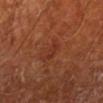Assessment: Part of a total-body skin-imaging series; this lesion was reviewed on a skin check and was not flagged for biopsy. Acquisition and patient details: Cropped from a total-body skin-imaging series; the visible field is about 15 mm. Automated image analysis of the tile measured a lesion–skin lightness drop of about 5 and a normalized border contrast of about 5. On the left lower leg. A male patient aged approximately 65. The tile uses cross-polarized illumination. The lesion's longest dimension is about 3 mm.